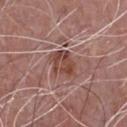* notes · catalogued during a skin exam; not biopsied